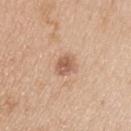Findings:
• biopsy status: catalogued during a skin exam; not biopsied
• patient: male, roughly 35 years of age
• image-analysis metrics: a nevus-likeness score of about 35/100 and a detector confidence of about 100 out of 100 that the crop contains a lesion
• illumination: white-light illumination
• anatomic site: the upper back
• image source: 15 mm crop, total-body photography